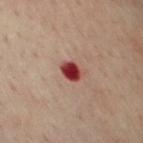– notes · total-body-photography surveillance lesion; no biopsy
– anatomic site · the chest
– patient · male, in their mid- to late 60s
– acquisition · total-body-photography crop, ~15 mm field of view
– automated lesion analysis · an average lesion color of about L≈38 a*≈33 b*≈24 (CIELAB); a classifier nevus-likeness of about 0/100